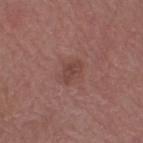{"biopsy_status": "not biopsied; imaged during a skin examination", "site": "left thigh", "automated_metrics": {"cielab_L": 43, "cielab_a": 21, "cielab_b": 23, "vs_skin_darker_L": 7.0, "vs_skin_contrast_norm": 6.0, "border_irregularity_0_10": 3.0, "peripheral_color_asymmetry": 0.5, "nevus_likeness_0_100": 5, "lesion_detection_confidence_0_100": 100}, "image": {"source": "total-body photography crop", "field_of_view_mm": 15}, "lesion_size": {"long_diameter_mm_approx": 3.0}, "patient": {"sex": "female", "age_approx": 70}}